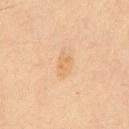Impression:
No biopsy was performed on this lesion — it was imaged during a full skin examination and was not determined to be concerning.
Acquisition and patient details:
The tile uses cross-polarized illumination. Located on the front of the torso. The patient is a male about 35 years old. Measured at roughly 3 mm in maximum diameter. Cropped from a whole-body photographic skin survey; the tile spans about 15 mm. Automated tile analysis of the lesion measured an area of roughly 5 mm². The analysis additionally found a mean CIELAB color near L≈62 a*≈17 b*≈36, about 6 CIELAB-L* units darker than the surrounding skin, and a normalized border contrast of about 5. It also reported border irregularity of about 2 on a 0–10 scale and internal color variation of about 2 on a 0–10 scale. The software also gave an automated nevus-likeness rating near 5 out of 100 and a detector confidence of about 100 out of 100 that the crop contains a lesion.